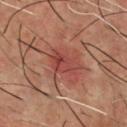Impression: The lesion was photographed on a routine skin check and not biopsied; there is no pathology result. Context: On the chest. Approximately 4 mm at its widest. Cropped from a total-body skin-imaging series; the visible field is about 15 mm. A male patient approximately 55 years of age. Imaged with cross-polarized lighting.